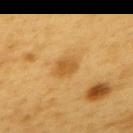| key | value |
|---|---|
| workup | total-body-photography surveillance lesion; no biopsy |
| anatomic site | the upper back |
| size | ~3.5 mm (longest diameter) |
| lighting | cross-polarized |
| patient | male, aged around 60 |
| imaging modality | ~15 mm tile from a whole-body skin photo |